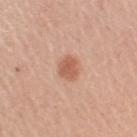The patient is a female about 65 years old. A lesion tile, about 15 mm wide, cut from a 3D total-body photograph. From the right upper arm. Measured at roughly 2.5 mm in maximum diameter.